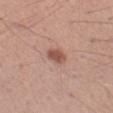biopsy_status: not biopsied; imaged during a skin examination
lesion_size:
  long_diameter_mm_approx: 2.5
lighting: white-light
image:
  source: total-body photography crop
  field_of_view_mm: 15
patient:
  sex: male
  age_approx: 45
site: left forearm